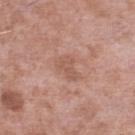lighting = white-light illumination; subject = male, roughly 55 years of age; body site = the left lower leg; acquisition = ~15 mm crop, total-body skin-cancer survey; size = ≈3 mm.